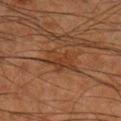- follow-up · catalogued during a skin exam; not biopsied
- lesion diameter · ≈4.5 mm
- patient · male, aged 63–67
- imaging modality · ~15 mm tile from a whole-body skin photo
- body site · the right lower leg
- lighting · cross-polarized illumination